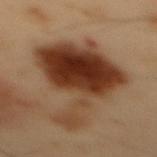| feature | finding |
|---|---|
| notes | catalogued during a skin exam; not biopsied |